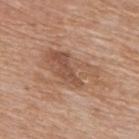{"biopsy_status": "not biopsied; imaged during a skin examination", "site": "mid back", "lighting": "white-light", "image": {"source": "total-body photography crop", "field_of_view_mm": 15}, "patient": {"sex": "male", "age_approx": 60}, "lesion_size": {"long_diameter_mm_approx": 7.0}}